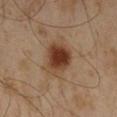The lesion was tiled from a total-body skin photograph and was not biopsied. This is a cross-polarized tile. The lesion-visualizer software estimated a lesion area of about 12 mm², an eccentricity of roughly 0.7, and a symmetry-axis asymmetry near 0.2. And it measured a mean CIELAB color near L≈35 a*≈18 b*≈28 and a normalized lesion–skin contrast near 11. It also reported border irregularity of about 2 on a 0–10 scale and internal color variation of about 5 on a 0–10 scale. And it measured a nevus-likeness score of about 100/100 and lesion-presence confidence of about 100/100. The lesion is on the left thigh. A 15 mm crop from a total-body photograph taken for skin-cancer surveillance. Longest diameter approximately 5 mm. A male subject roughly 60 years of age.